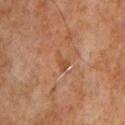Recorded during total-body skin imaging; not selected for excision or biopsy.
The lesion-visualizer software estimated a footprint of about 2.5 mm² and a symmetry-axis asymmetry near 0.6. The software also gave an average lesion color of about L≈44 a*≈21 b*≈33 (CIELAB), about 6 CIELAB-L* units darker than the surrounding skin, and a normalized lesion–skin contrast near 6. The software also gave a border-irregularity rating of about 6/10.
Longest diameter approximately 2.5 mm.
The lesion is on the upper back.
A lesion tile, about 15 mm wide, cut from a 3D total-body photograph.
The subject is a male aged approximately 60.